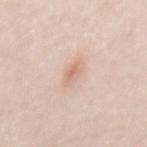Imaged during a routine full-body skin examination; the lesion was not biopsied and no histopathology is available.
A male subject, about 35 years old.
From the back.
This is a white-light tile.
About 3 mm across.
A region of skin cropped from a whole-body photographic capture, roughly 15 mm wide.
The lesion-visualizer software estimated a lesion area of about 4 mm², an outline eccentricity of about 0.85 (0 = round, 1 = elongated), and a shape-asymmetry score of about 0.3 (0 = symmetric). The analysis additionally found a mean CIELAB color near L≈69 a*≈19 b*≈28 and roughly 8 lightness units darker than nearby skin. And it measured a border-irregularity rating of about 3/10 and a color-variation rating of about 3.5/10. The software also gave lesion-presence confidence of about 100/100.